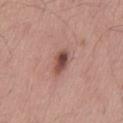– workup: imaged on a skin check; not biopsied
– anatomic site: the back
– diameter: ~3 mm (longest diameter)
– illumination: white-light
– patient: male, about 55 years old
– image: ~15 mm crop, total-body skin-cancer survey